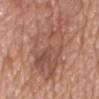notes = catalogued during a skin exam; not biopsied | imaging modality = ~15 mm crop, total-body skin-cancer survey | location = the chest | subject = male, aged 78–82.